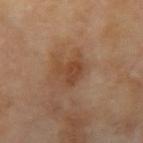lesion size — ~3.5 mm (longest diameter); body site — the right upper arm; lighting — cross-polarized illumination; patient — male, in their 70s; image source — ~15 mm tile from a whole-body skin photo.